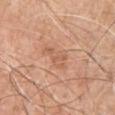Impression:
No biopsy was performed on this lesion — it was imaged during a full skin examination and was not determined to be concerning.
Context:
Longest diameter approximately 3.5 mm. A close-up tile cropped from a whole-body skin photograph, about 15 mm across. Imaged with white-light lighting. The total-body-photography lesion software estimated an outline eccentricity of about 0.85 (0 = round, 1 = elongated). The software also gave a border-irregularity rating of about 4.5/10, a color-variation rating of about 2/10, and a peripheral color-asymmetry measure near 0.5. A male subject aged approximately 60. On the chest.